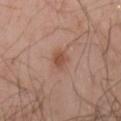<tbp_lesion>
<biopsy_status>not biopsied; imaged during a skin examination</biopsy_status>
<patient>
  <sex>male</sex>
  <age_approx>45</age_approx>
</patient>
<lighting>cross-polarized</lighting>
<lesion_size>
  <long_diameter_mm_approx>2.5</long_diameter_mm_approx>
</lesion_size>
<image>
  <source>total-body photography crop</source>
  <field_of_view_mm>15</field_of_view_mm>
</image>
<site>right forearm</site>
</tbp_lesion>